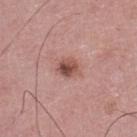A close-up tile cropped from a whole-body skin photograph, about 15 mm across. Automated tile analysis of the lesion measured a lesion area of about 4.5 mm² and an eccentricity of roughly 0.5. The software also gave an automated nevus-likeness rating near 85 out of 100 and a lesion-detection confidence of about 100/100. From the left lower leg. Longest diameter approximately 2.5 mm. The patient is a male roughly 75 years of age.